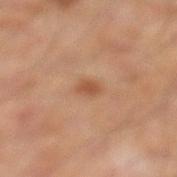workup: no biopsy performed (imaged during a skin exam)
acquisition: total-body-photography crop, ~15 mm field of view
tile lighting: cross-polarized
TBP lesion metrics: a mean CIELAB color near L≈48 a*≈22 b*≈32, a lesion–skin lightness drop of about 9, and a normalized lesion–skin contrast near 7; a border-irregularity index near 2.5/10, a color-variation rating of about 1/10, and radial color variation of about 0.5; a nevus-likeness score of about 85/100 and a lesion-detection confidence of about 100/100
patient: male, aged 53–57
lesion diameter: ≈2 mm
body site: the left lower leg